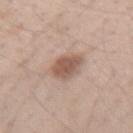Assessment: No biopsy was performed on this lesion — it was imaged during a full skin examination and was not determined to be concerning. Context: Approximately 3 mm at its widest. Captured under white-light illumination. The subject is a female aged around 40. Cropped from a whole-body photographic skin survey; the tile spans about 15 mm. The lesion is on the right forearm. Automated tile analysis of the lesion measured a lesion area of about 7.5 mm² and an outline eccentricity of about 0.4 (0 = round, 1 = elongated). And it measured a lesion color around L≈55 a*≈18 b*≈26 in CIELAB. The analysis additionally found a classifier nevus-likeness of about 90/100.